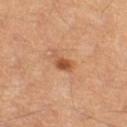Recorded during total-body skin imaging; not selected for excision or biopsy.
From the right thigh.
Cropped from a whole-body photographic skin survey; the tile spans about 15 mm.
A female patient in their 60s.